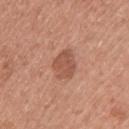The lesion was tiled from a total-body skin photograph and was not biopsied.